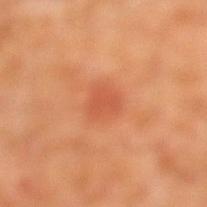Impression:
Captured during whole-body skin photography for melanoma surveillance; the lesion was not biopsied.
Context:
The lesion-visualizer software estimated a classifier nevus-likeness of about 5/100 and a lesion-detection confidence of about 100/100. The lesion is on the left lower leg. A close-up tile cropped from a whole-body skin photograph, about 15 mm across. A male subject, aged 28–32.